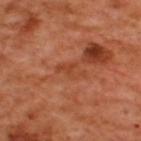anatomic site: the upper back; acquisition: total-body-photography crop, ~15 mm field of view; subject: male, aged approximately 50.